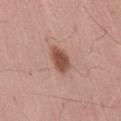The lesion was tiled from a total-body skin photograph and was not biopsied. The lesion is located on the lower back. This is a white-light tile. The recorded lesion diameter is about 3.5 mm. A male subject, aged 53 to 57. A roughly 15 mm field-of-view crop from a total-body skin photograph. The total-body-photography lesion software estimated internal color variation of about 2 on a 0–10 scale and radial color variation of about 0.5. The analysis additionally found an automated nevus-likeness rating near 100 out of 100.